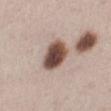Clinical impression: Imaged during a routine full-body skin examination; the lesion was not biopsied and no histopathology is available. Background: A female patient, approximately 40 years of age. This image is a 15 mm lesion crop taken from a total-body photograph. Measured at roughly 4 mm in maximum diameter. The tile uses white-light illumination. The lesion is on the left lower leg.